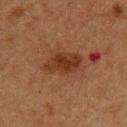Imaged during a routine full-body skin examination; the lesion was not biopsied and no histopathology is available. A female subject, about 55 years old. The lesion's longest dimension is about 4.5 mm. Located on the upper back. The tile uses cross-polarized illumination. Cropped from a whole-body photographic skin survey; the tile spans about 15 mm. Automated tile analysis of the lesion measured an average lesion color of about L≈28 a*≈19 b*≈27 (CIELAB), about 8 CIELAB-L* units darker than the surrounding skin, and a lesion-to-skin contrast of about 8.5 (normalized; higher = more distinct). It also reported an automated nevus-likeness rating near 5 out of 100.